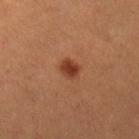Imaged during a routine full-body skin examination; the lesion was not biopsied and no histopathology is available. A 15 mm close-up extracted from a 3D total-body photography capture. Located on the right lower leg. A female subject, in their mid-20s. Measured at roughly 2.5 mm in maximum diameter. Captured under cross-polarized illumination.